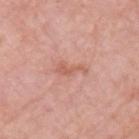The lesion was photographed on a routine skin check and not biopsied; there is no pathology result. Automated tile analysis of the lesion measured a footprint of about 3 mm². The analysis additionally found a lesion color around L≈59 a*≈26 b*≈30 in CIELAB and a normalized border contrast of about 6. The software also gave an automated nevus-likeness rating near 0 out of 100. On the arm. A close-up tile cropped from a whole-body skin photograph, about 15 mm across. A female patient aged around 65. The recorded lesion diameter is about 3.5 mm.